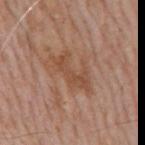On the mid back.
A male subject, aged 58 to 62.
A 15 mm crop from a total-body photograph taken for skin-cancer surveillance.